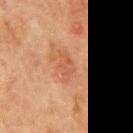biopsy status: imaged on a skin check; not biopsied | imaging modality: ~15 mm tile from a whole-body skin photo | patient: male, aged 68–72 | lighting: cross-polarized illumination | location: the mid back | lesion diameter: ~3 mm (longest diameter).